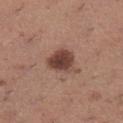notes: total-body-photography surveillance lesion; no biopsy | patient: female, approximately 30 years of age | location: the left lower leg | illumination: white-light illumination | diameter: about 3.5 mm | image source: total-body-photography crop, ~15 mm field of view.